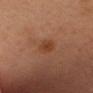Captured during whole-body skin photography for melanoma surveillance; the lesion was not biopsied.